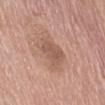Captured during whole-body skin photography for melanoma surveillance; the lesion was not biopsied.
From the abdomen.
About 3 mm across.
A roughly 15 mm field-of-view crop from a total-body skin photograph.
The subject is a female approximately 75 years of age.
An algorithmic analysis of the crop reported an average lesion color of about L≈54 a*≈20 b*≈27 (CIELAB) and a lesion–skin lightness drop of about 8. The analysis additionally found a border-irregularity index near 2.5/10, a within-lesion color-variation index near 1.5/10, and peripheral color asymmetry of about 0.5.
This is a white-light tile.